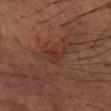notes = imaged on a skin check; not biopsied | imaging modality = total-body-photography crop, ~15 mm field of view | site = the arm | subject = male, aged approximately 65.